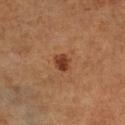Recorded during total-body skin imaging; not selected for excision or biopsy. Captured under cross-polarized illumination. About 2.5 mm across. The lesion is on the right lower leg. A 15 mm close-up tile from a total-body photography series done for melanoma screening. The patient is aged 68–72. The lesion-visualizer software estimated peripheral color asymmetry of about 1. It also reported a classifier nevus-likeness of about 95/100 and a lesion-detection confidence of about 100/100.